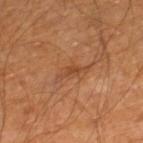workup: no biopsy performed (imaged during a skin exam) | patient: male, approximately 60 years of age | body site: the right lower leg | image: 15 mm crop, total-body photography.